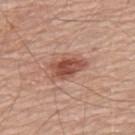Recorded during total-body skin imaging; not selected for excision or biopsy. Cropped from a total-body skin-imaging series; the visible field is about 15 mm. A male subject, aged approximately 70. The lesion is located on the upper back. The tile uses white-light illumination. About 4.5 mm across.